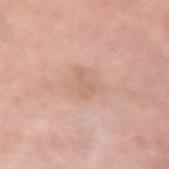Impression: Captured during whole-body skin photography for melanoma surveillance; the lesion was not biopsied. Acquisition and patient details: A region of skin cropped from a whole-body photographic capture, roughly 15 mm wide. The lesion is located on the left forearm. This is a white-light tile. A female subject in their 60s. About 3 mm across.